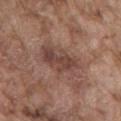Q: Is there a histopathology result?
A: catalogued during a skin exam; not biopsied
Q: Automated lesion metrics?
A: an outline eccentricity of about 0.85 (0 = round, 1 = elongated) and a symmetry-axis asymmetry near 0.2; an average lesion color of about L≈45 a*≈19 b*≈24 (CIELAB), roughly 8 lightness units darker than nearby skin, and a normalized border contrast of about 6.5; a border-irregularity rating of about 3.5/10, a within-lesion color-variation index near 4.5/10, and radial color variation of about 1.5; a nevus-likeness score of about 0/100 and a lesion-detection confidence of about 95/100
Q: Who is the patient?
A: male, about 75 years old
Q: Where on the body is the lesion?
A: the mid back
Q: What is the lesion's diameter?
A: ≈6.5 mm
Q: What kind of image is this?
A: ~15 mm tile from a whole-body skin photo